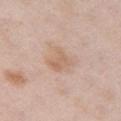Findings:
* notes · imaged on a skin check; not biopsied
* anatomic site · the chest
* lesion size · ~3.5 mm (longest diameter)
* automated metrics · a footprint of about 7.5 mm² and an eccentricity of roughly 0.55; border irregularity of about 3.5 on a 0–10 scale and peripheral color asymmetry of about 1
* imaging modality · ~15 mm tile from a whole-body skin photo
* subject · female, roughly 65 years of age